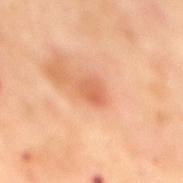Clinical impression:
Part of a total-body skin-imaging series; this lesion was reviewed on a skin check and was not flagged for biopsy.
Acquisition and patient details:
The subject is a female aged 53 to 57. A 15 mm close-up tile from a total-body photography series done for melanoma screening. The recorded lesion diameter is about 2.5 mm. Imaged with cross-polarized lighting. The lesion is on the mid back.